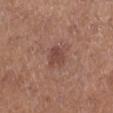Impression:
The lesion was tiled from a total-body skin photograph and was not biopsied.
Background:
Automated image analysis of the tile measured a border-irregularity index near 2.5/10 and a color-variation rating of about 2/10. It also reported an automated nevus-likeness rating near 40 out of 100 and a detector confidence of about 100 out of 100 that the crop contains a lesion. A female subject, about 65 years old. The lesion is on the left leg. Captured under white-light illumination. A lesion tile, about 15 mm wide, cut from a 3D total-body photograph.